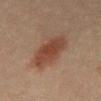notes: total-body-photography surveillance lesion; no biopsy
lesion size: about 6.5 mm
acquisition: ~15 mm tile from a whole-body skin photo
illumination: cross-polarized illumination
automated metrics: a lesion area of about 16 mm² and a symmetry-axis asymmetry near 0.15; a mean CIELAB color near L≈35 a*≈18 b*≈24, about 9 CIELAB-L* units darker than the surrounding skin, and a normalized lesion–skin contrast near 8.5; lesion-presence confidence of about 100/100
patient: male, aged 58 to 62
body site: the back